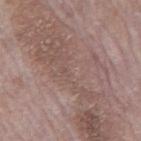automated metrics = an eccentricity of roughly 0.95 and two-axis asymmetry of about 0.4; a border-irregularity index near 8/10, a color-variation rating of about 5.5/10, and peripheral color asymmetry of about 2 | imaging modality = ~15 mm crop, total-body skin-cancer survey | location = the back | lighting = white-light | lesion size = ~20.5 mm (longest diameter) | patient = male, aged 68 to 72.